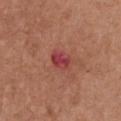Assessment:
The lesion was tiled from a total-body skin photograph and was not biopsied.
Clinical summary:
The subject is a female approximately 65 years of age. On the chest. This image is a 15 mm lesion crop taken from a total-body photograph.